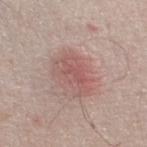Cropped from a total-body skin-imaging series; the visible field is about 15 mm.
From the left thigh.
The patient is a male aged 68 to 72.